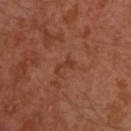No biopsy was performed on this lesion — it was imaged during a full skin examination and was not determined to be concerning.
Imaged with cross-polarized lighting.
A male subject in their 30s.
About 2.5 mm across.
A 15 mm close-up tile from a total-body photography series done for melanoma screening.
An algorithmic analysis of the crop reported a lesion color around L≈36 a*≈25 b*≈31 in CIELAB, a lesion–skin lightness drop of about 6, and a lesion-to-skin contrast of about 6 (normalized; higher = more distinct). And it measured an automated nevus-likeness rating near 0 out of 100.
From the left upper arm.